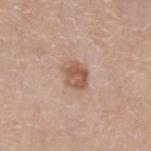No biopsy was performed on this lesion — it was imaged during a full skin examination and was not determined to be concerning. Cropped from a total-body skin-imaging series; the visible field is about 15 mm. From the right lower leg. The patient is a female roughly 75 years of age.